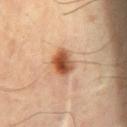Impression:
The lesion was photographed on a routine skin check and not biopsied; there is no pathology result.
Context:
From the mid back. A close-up tile cropped from a whole-body skin photograph, about 15 mm across. The lesion's longest dimension is about 3.5 mm. A male subject about 50 years old.